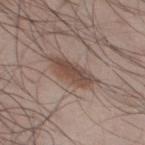Clinical impression: The lesion was photographed on a routine skin check and not biopsied; there is no pathology result. Acquisition and patient details: A region of skin cropped from a whole-body photographic capture, roughly 15 mm wide. A male patient, in their mid-40s. The lesion is located on the upper back.